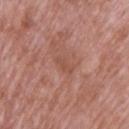workup — catalogued during a skin exam; not biopsied | lesion size — ≈3 mm | subject — male, aged approximately 70 | site — the upper back | image source — total-body-photography crop, ~15 mm field of view | illumination — white-light.